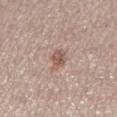workup = no biopsy performed (imaged during a skin exam) | anatomic site = the left lower leg | image = ~15 mm tile from a whole-body skin photo | subject = female, aged 48–52.